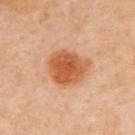| key | value |
|---|---|
| follow-up | catalogued during a skin exam; not biopsied |
| subject | female, in their mid-40s |
| body site | the upper back |
| lesion diameter | about 4.5 mm |
| tile lighting | cross-polarized illumination |
| image | 15 mm crop, total-body photography |